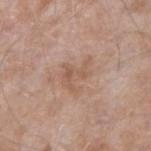Case summary:
• biopsy status · imaged on a skin check; not biopsied
• site · the right forearm
• lesion size · ~4 mm (longest diameter)
• subject · male, aged 73 to 77
• TBP lesion metrics · an average lesion color of about L≈56 a*≈19 b*≈28 (CIELAB), roughly 7 lightness units darker than nearby skin, and a normalized lesion–skin contrast near 5
• imaging modality · ~15 mm crop, total-body skin-cancer survey
• illumination · white-light illumination